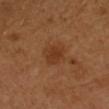Captured during whole-body skin photography for melanoma surveillance; the lesion was not biopsied.
A female patient, aged around 50.
The recorded lesion diameter is about 3.5 mm.
A roughly 15 mm field-of-view crop from a total-body skin photograph.
The lesion is on the left upper arm.
Captured under cross-polarized illumination.